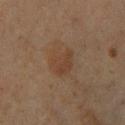Q: Who is the patient?
A: male, aged approximately 60
Q: Lesion location?
A: the right lower leg
Q: How was this image acquired?
A: 15 mm crop, total-body photography